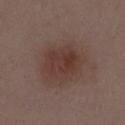biopsy_status: not biopsied; imaged during a skin examination
lighting: white-light
automated_metrics:
  cielab_L: 36
  cielab_a: 18
  cielab_b: 22
  border_irregularity_0_10: 2.0
  color_variation_0_10: 3.5
  peripheral_color_asymmetry: 1.5
lesion_size:
  long_diameter_mm_approx: 4.5
patient:
  sex: female
  age_approx: 30
image:
  source: total-body photography crop
  field_of_view_mm: 15
site: mid back